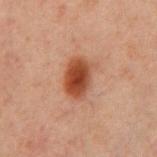The lesion was photographed on a routine skin check and not biopsied; there is no pathology result. A 15 mm close-up extracted from a 3D total-body photography capture. Captured under cross-polarized illumination. A male subject, aged 58–62. Measured at roughly 4.5 mm in maximum diameter. Located on the front of the torso.